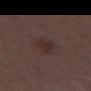Imaged during a routine full-body skin examination; the lesion was not biopsied and no histopathology is available.
A male subject, aged 68–72.
Located on the left thigh.
A 15 mm crop from a total-body photograph taken for skin-cancer surveillance.